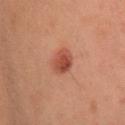Part of a total-body skin-imaging series; this lesion was reviewed on a skin check and was not flagged for biopsy. The lesion's longest dimension is about 3.5 mm. A lesion tile, about 15 mm wide, cut from a 3D total-body photograph. Captured under cross-polarized illumination. Located on the head or neck. Automated image analysis of the tile measured an eccentricity of roughly 0.75 and a shape-asymmetry score of about 0.2 (0 = symmetric). The analysis additionally found a lesion color around L≈40 a*≈25 b*≈27 in CIELAB, a lesion–skin lightness drop of about 10, and a normalized lesion–skin contrast near 8. And it measured a border-irregularity index near 1.5/10, a color-variation rating of about 3/10, and radial color variation of about 1. A female patient aged approximately 55.